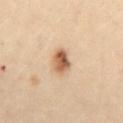Assessment:
Captured during whole-body skin photography for melanoma surveillance; the lesion was not biopsied.
Context:
A female subject, about 30 years old. The recorded lesion diameter is about 3 mm. The lesion is on the abdomen. A 15 mm close-up tile from a total-body photography series done for melanoma screening. Imaged with cross-polarized lighting.